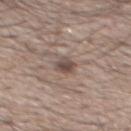Assessment:
The lesion was tiled from a total-body skin photograph and was not biopsied.
Background:
The lesion's longest dimension is about 2.5 mm. A close-up tile cropped from a whole-body skin photograph, about 15 mm across. From the mid back. A male patient aged 63–67.